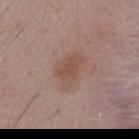No biopsy was performed on this lesion — it was imaged during a full skin examination and was not determined to be concerning.
Located on the mid back.
A male subject about 55 years old.
A roughly 15 mm field-of-view crop from a total-body skin photograph.
Automated image analysis of the tile measured a footprint of about 8.5 mm², an eccentricity of roughly 0.8, and two-axis asymmetry of about 0.3.